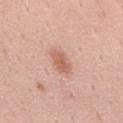Recorded during total-body skin imaging; not selected for excision or biopsy. This image is a 15 mm lesion crop taken from a total-body photograph. This is a white-light tile. Measured at roughly 3.5 mm in maximum diameter. Located on the mid back. A male patient in their mid-50s.